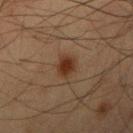Part of a total-body skin-imaging series; this lesion was reviewed on a skin check and was not flagged for biopsy. A lesion tile, about 15 mm wide, cut from a 3D total-body photograph. From the left upper arm. Captured under cross-polarized illumination. The lesion's longest dimension is about 2.5 mm. The patient is a male roughly 55 years of age. An algorithmic analysis of the crop reported a footprint of about 4.5 mm², an eccentricity of roughly 0.45, and a symmetry-axis asymmetry near 0.2. The software also gave an average lesion color of about L≈28 a*≈18 b*≈26 (CIELAB), about 10 CIELAB-L* units darker than the surrounding skin, and a lesion-to-skin contrast of about 10.5 (normalized; higher = more distinct). And it measured internal color variation of about 2 on a 0–10 scale and a peripheral color-asymmetry measure near 0.5.